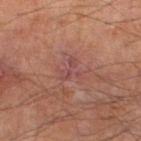biopsy status: catalogued during a skin exam; not biopsied
image source: ~15 mm tile from a whole-body skin photo
illumination: cross-polarized illumination
diameter: about 3.5 mm
site: the left lower leg
patient: male, aged around 65
automated metrics: an outline eccentricity of about 0.35 (0 = round, 1 = elongated); a border-irregularity index near 7.5/10, a within-lesion color-variation index near 2/10, and radial color variation of about 0.5; a nevus-likeness score of about 0/100 and a detector confidence of about 90 out of 100 that the crop contains a lesion